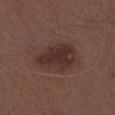Part of a total-body skin-imaging series; this lesion was reviewed on a skin check and was not flagged for biopsy.